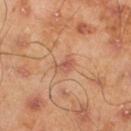notes: no biopsy performed (imaged during a skin exam) | lesion size: ~2.5 mm (longest diameter) | image: total-body-photography crop, ~15 mm field of view | subject: male, aged around 45 | automated lesion analysis: an area of roughly 3 mm²; border irregularity of about 3.5 on a 0–10 scale, a color-variation rating of about 1.5/10, and radial color variation of about 0.5; a classifier nevus-likeness of about 0/100 and lesion-presence confidence of about 100/100 | anatomic site: the left lower leg | tile lighting: cross-polarized.